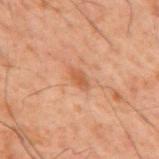workup: total-body-photography surveillance lesion; no biopsy
subject: male, roughly 60 years of age
site: the mid back
image: 15 mm crop, total-body photography